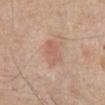{
  "biopsy_status": "not biopsied; imaged during a skin examination",
  "lighting": "white-light",
  "image": {
    "source": "total-body photography crop",
    "field_of_view_mm": 15
  },
  "lesion_size": {
    "long_diameter_mm_approx": 3.5
  },
  "automated_metrics": {
    "area_mm2_approx": 5.0,
    "eccentricity": 0.85,
    "shape_asymmetry": 0.4,
    "nevus_likeness_0_100": 65,
    "lesion_detection_confidence_0_100": 100
  },
  "site": "front of the torso",
  "patient": {
    "sex": "male",
    "age_approx": 80
  }
}